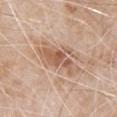Part of a total-body skin-imaging series; this lesion was reviewed on a skin check and was not flagged for biopsy. The subject is a male in their 80s. From the chest. A lesion tile, about 15 mm wide, cut from a 3D total-body photograph.